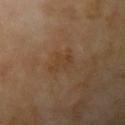Impression: No biopsy was performed on this lesion — it was imaged during a full skin examination and was not determined to be concerning. Background: A close-up tile cropped from a whole-body skin photograph, about 15 mm across. This is a cross-polarized tile. Located on the left upper arm. A male patient aged 68 to 72. About 3 mm across.